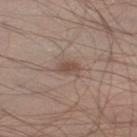Clinical impression:
This lesion was catalogued during total-body skin photography and was not selected for biopsy.
Background:
An algorithmic analysis of the crop reported an average lesion color of about L≈50 a*≈16 b*≈25 (CIELAB), roughly 8 lightness units darker than nearby skin, and a normalized border contrast of about 6.5. The recorded lesion diameter is about 2.5 mm. The lesion is located on the left thigh. A male patient, aged 53 to 57. A lesion tile, about 15 mm wide, cut from a 3D total-body photograph. Captured under white-light illumination.